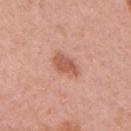Part of a total-body skin-imaging series; this lesion was reviewed on a skin check and was not flagged for biopsy.
Measured at roughly 3.5 mm in maximum diameter.
A 15 mm crop from a total-body photograph taken for skin-cancer surveillance.
Located on the right upper arm.
The subject is a female approximately 40 years of age.
The tile uses white-light illumination.
The lesion-visualizer software estimated border irregularity of about 2 on a 0–10 scale, internal color variation of about 2 on a 0–10 scale, and peripheral color asymmetry of about 0.5.